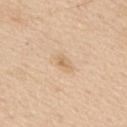lighting: white-light illumination
subject: male, roughly 60 years of age
location: the upper back
diameter: ≈2.5 mm
acquisition: ~15 mm tile from a whole-body skin photo